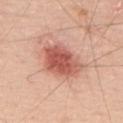Impression: No biopsy was performed on this lesion — it was imaged during a full skin examination and was not determined to be concerning. Image and clinical context: Located on the upper back. The patient is a male aged 68 to 72. Cropped from a whole-body photographic skin survey; the tile spans about 15 mm.